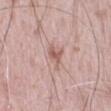Assessment:
This lesion was catalogued during total-body skin photography and was not selected for biopsy.
Clinical summary:
The lesion is located on the abdomen. Measured at roughly 3.5 mm in maximum diameter. This is a white-light tile. A lesion tile, about 15 mm wide, cut from a 3D total-body photograph. A male patient approximately 80 years of age.